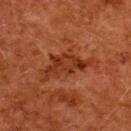Approximately 6 mm at its widest. The lesion is located on the upper back. Captured under cross-polarized illumination. The patient is a female aged 48–52. The lesion-visualizer software estimated a border-irregularity index near 6.5/10 and a peripheral color-asymmetry measure near 1.5. The analysis additionally found an automated nevus-likeness rating near 0 out of 100 and lesion-presence confidence of about 100/100. Cropped from a whole-body photographic skin survey; the tile spans about 15 mm.